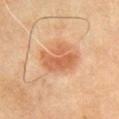biopsy status = catalogued during a skin exam; not biopsied | subject = male, aged around 65 | location = the chest | image source = ~15 mm tile from a whole-body skin photo.